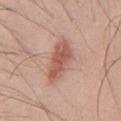notes = imaged on a skin check; not biopsied | patient = male, approximately 45 years of age | lesion diameter = ~5.5 mm (longest diameter) | lighting = white-light illumination | image source = ~15 mm tile from a whole-body skin photo | location = the chest.